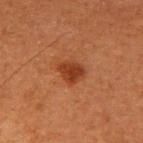biopsy status: no biopsy performed (imaged during a skin exam)
body site: the right upper arm
acquisition: ~15 mm crop, total-body skin-cancer survey
lighting: cross-polarized
lesion size: ≈3 mm
automated metrics: a lesion color around L≈32 a*≈24 b*≈31 in CIELAB, about 9 CIELAB-L* units darker than the surrounding skin, and a lesion-to-skin contrast of about 8.5 (normalized; higher = more distinct); a border-irregularity rating of about 2/10 and peripheral color asymmetry of about 0.5
subject: male, aged around 75